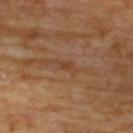Imaged during a routine full-body skin examination; the lesion was not biopsied and no histopathology is available. This is a cross-polarized tile. A roughly 15 mm field-of-view crop from a total-body skin photograph. The patient is a male aged around 70. Automated image analysis of the tile measured an area of roughly 3.5 mm², an outline eccentricity of about 0.85 (0 = round, 1 = elongated), and two-axis asymmetry of about 0.4. The analysis additionally found a lesion color around L≈41 a*≈20 b*≈31 in CIELAB, about 6 CIELAB-L* units darker than the surrounding skin, and a lesion-to-skin contrast of about 5.5 (normalized; higher = more distinct). It also reported a border-irregularity index near 4.5/10, a within-lesion color-variation index near 0/10, and peripheral color asymmetry of about 0. It also reported a classifier nevus-likeness of about 0/100 and a detector confidence of about 100 out of 100 that the crop contains a lesion. The recorded lesion diameter is about 3 mm. From the upper back.